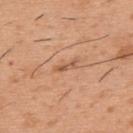<tbp_lesion>
  <biopsy_status>not biopsied; imaged during a skin examination</biopsy_status>
  <image>
    <source>total-body photography crop</source>
    <field_of_view_mm>15</field_of_view_mm>
  </image>
  <site>upper back</site>
  <patient>
    <sex>male</sex>
    <age_approx>40</age_approx>
  </patient>
</tbp_lesion>